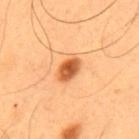{
  "site": "upper back",
  "patient": {
    "sex": "male",
    "age_approx": 55
  },
  "image": {
    "source": "total-body photography crop",
    "field_of_view_mm": 15
  }
}